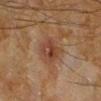Q: Was a biopsy performed?
A: no biopsy performed (imaged during a skin exam)
Q: How was this image acquired?
A: ~15 mm tile from a whole-body skin photo
Q: Lesion location?
A: the leg
Q: Patient demographics?
A: male, aged 63–67
Q: What did automated image analysis measure?
A: an area of roughly 9.5 mm², an outline eccentricity of about 0.45 (0 = round, 1 = elongated), and a shape-asymmetry score of about 0.2 (0 = symmetric)
Q: How large is the lesion?
A: about 4 mm
Q: How was the tile lit?
A: cross-polarized illumination